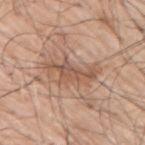Q: Was a biopsy performed?
A: catalogued during a skin exam; not biopsied
Q: What is the anatomic site?
A: the upper back
Q: What are the patient's age and sex?
A: male, roughly 70 years of age
Q: Illumination type?
A: white-light illumination
Q: What did automated image analysis measure?
A: a lesion color around L≈55 a*≈19 b*≈30 in CIELAB and a normalized border contrast of about 7; a border-irregularity index near 7/10; an automated nevus-likeness rating near 0 out of 100 and a detector confidence of about 95 out of 100 that the crop contains a lesion
Q: What is the imaging modality?
A: total-body-photography crop, ~15 mm field of view